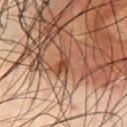The lesion was photographed on a routine skin check and not biopsied; there is no pathology result.
The lesion is located on the chest.
Measured at roughly 2.5 mm in maximum diameter.
The patient is a male aged 53 to 57.
A 15 mm close-up tile from a total-body photography series done for melanoma screening.